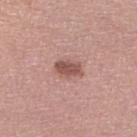This lesion was catalogued during total-body skin photography and was not selected for biopsy. Measured at roughly 3 mm in maximum diameter. This is a white-light tile. This image is a 15 mm lesion crop taken from a total-body photograph. From the leg. A female patient aged 58 to 62. Automated image analysis of the tile measured a mean CIELAB color near L≈53 a*≈22 b*≈24, about 12 CIELAB-L* units darker than the surrounding skin, and a normalized border contrast of about 8. The software also gave border irregularity of about 2 on a 0–10 scale and a within-lesion color-variation index near 2.5/10.